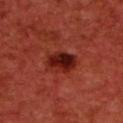Recorded during total-body skin imaging; not selected for excision or biopsy. A 15 mm close-up extracted from a 3D total-body photography capture. Longest diameter approximately 3.5 mm. A male subject aged 58 to 62. The lesion is located on the upper back.